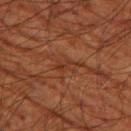The lesion was photographed on a routine skin check and not biopsied; there is no pathology result. A lesion tile, about 15 mm wide, cut from a 3D total-body photograph. The subject is a male aged 78 to 82. From the left thigh.